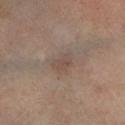Part of a total-body skin-imaging series; this lesion was reviewed on a skin check and was not flagged for biopsy. A male patient aged 63 to 67. An algorithmic analysis of the crop reported a lesion area of about 5.5 mm². And it measured a mean CIELAB color near L≈46 a*≈14 b*≈21, roughly 6 lightness units darker than nearby skin, and a normalized border contrast of about 5. It also reported an automated nevus-likeness rating near 0 out of 100 and a lesion-detection confidence of about 100/100. A 15 mm close-up tile from a total-body photography series done for melanoma screening. About 3 mm across. The tile uses cross-polarized illumination. On the leg.